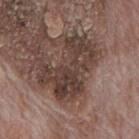Q: Was a biopsy performed?
A: imaged on a skin check; not biopsied
Q: Lesion location?
A: the mid back
Q: Automated lesion metrics?
A: a mean CIELAB color near L≈38 a*≈16 b*≈21, a lesion–skin lightness drop of about 10, and a normalized lesion–skin contrast near 9; a border-irregularity rating of about 9.5/10, a within-lesion color-variation index near 5.5/10, and peripheral color asymmetry of about 2; a nevus-likeness score of about 5/100 and a lesion-detection confidence of about 90/100
Q: What are the patient's age and sex?
A: male, in their 70s
Q: Illumination type?
A: white-light illumination
Q: What is the imaging modality?
A: 15 mm crop, total-body photography
Q: Lesion size?
A: ≈7 mm